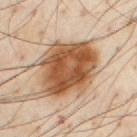The lesion was photographed on a routine skin check and not biopsied; there is no pathology result.
A region of skin cropped from a whole-body photographic capture, roughly 15 mm wide.
Approximately 8 mm at its widest.
The subject is a male aged around 55.
The lesion is located on the chest.
Captured under cross-polarized illumination.